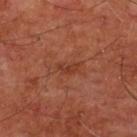Imaged during a routine full-body skin examination; the lesion was not biopsied and no histopathology is available. Measured at roughly 2.5 mm in maximum diameter. A roughly 15 mm field-of-view crop from a total-body skin photograph. Automated image analysis of the tile measured an area of roughly 3 mm² and an eccentricity of roughly 0.85. The software also gave a lesion color around L≈33 a*≈25 b*≈29 in CIELAB, a lesion–skin lightness drop of about 6, and a normalized lesion–skin contrast near 6. And it measured a border-irregularity rating of about 4.5/10, internal color variation of about 0 on a 0–10 scale, and a peripheral color-asymmetry measure near 0. A male patient, aged approximately 60. The tile uses cross-polarized illumination. Located on the chest.